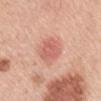<lesion>
  <biopsy_status>not biopsied; imaged during a skin examination</biopsy_status>
  <image>
    <source>total-body photography crop</source>
    <field_of_view_mm>15</field_of_view_mm>
  </image>
  <site>mid back</site>
  <automated_metrics>
    <cielab_L>62</cielab_L>
    <cielab_a>28</cielab_a>
    <cielab_b>28</cielab_b>
    <vs_skin_darker_L>10.0</vs_skin_darker_L>
    <color_variation_0_10>2.5</color_variation_0_10>
    <nevus_likeness_0_100>95</nevus_likeness_0_100>
    <lesion_detection_confidence_0_100>100</lesion_detection_confidence_0_100>
  </automated_metrics>
  <lesion_size>
    <long_diameter_mm_approx>4.0</long_diameter_mm_approx>
  </lesion_size>
  <patient>
    <sex>female</sex>
    <age_approx>65</age_approx>
  </patient>
</lesion>